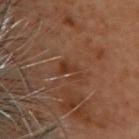Q: Is there a histopathology result?
A: imaged on a skin check; not biopsied
Q: What did automated image analysis measure?
A: a footprint of about 4 mm², a shape eccentricity near 0.8, and a symmetry-axis asymmetry near 0.55; a mean CIELAB color near L≈30 a*≈19 b*≈27, about 5 CIELAB-L* units darker than the surrounding skin, and a lesion-to-skin contrast of about 6.5 (normalized; higher = more distinct)
Q: What is the anatomic site?
A: the upper back
Q: How was the tile lit?
A: cross-polarized illumination
Q: What kind of image is this?
A: ~15 mm tile from a whole-body skin photo
Q: What are the patient's age and sex?
A: female, in their 60s